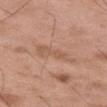notes: no biopsy performed (imaged during a skin exam)
diameter: ≈5 mm
illumination: white-light illumination
patient: male, aged approximately 50
image: 15 mm crop, total-body photography
automated metrics: a lesion area of about 7 mm², an outline eccentricity of about 0.95 (0 = round, 1 = elongated), and two-axis asymmetry of about 0.35; a lesion color around L≈57 a*≈21 b*≈30 in CIELAB, roughly 6 lightness units darker than nearby skin, and a normalized border contrast of about 4.5; border irregularity of about 6 on a 0–10 scale, a within-lesion color-variation index near 1.5/10, and peripheral color asymmetry of about 0.5
site: the left thigh